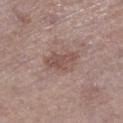Assessment: This lesion was catalogued during total-body skin photography and was not selected for biopsy. Acquisition and patient details: Captured under white-light illumination. A lesion tile, about 15 mm wide, cut from a 3D total-body photograph. A female subject aged around 65. From the right lower leg.